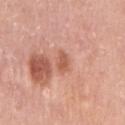location: the right upper arm | diameter: ≈2.5 mm | patient: female, aged around 40 | imaging modality: 15 mm crop, total-body photography.